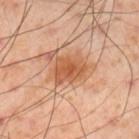Q: Was this lesion biopsied?
A: no biopsy performed (imaged during a skin exam)
Q: What kind of image is this?
A: 15 mm crop, total-body photography
Q: Where on the body is the lesion?
A: the right lower leg
Q: Patient demographics?
A: male, approximately 55 years of age